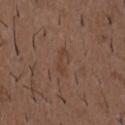Impression: Recorded during total-body skin imaging; not selected for excision or biopsy. Context: A male subject, roughly 50 years of age. This is a white-light tile. The lesion's longest dimension is about 3.5 mm. A lesion tile, about 15 mm wide, cut from a 3D total-body photograph. The lesion is on the front of the torso.